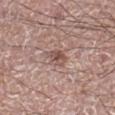follow-up=catalogued during a skin exam; not biopsied
acquisition=total-body-photography crop, ~15 mm field of view
size=about 3 mm
location=the right lower leg
subject=male, about 60 years old